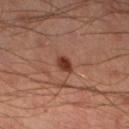workup=catalogued during a skin exam; not biopsied | subject=male, aged 43–47 | diameter=~2 mm (longest diameter) | location=the left lower leg | image source=15 mm crop, total-body photography | illumination=cross-polarized illumination | TBP lesion metrics=an area of roughly 3 mm² and a shape eccentricity near 0.6; a mean CIELAB color near L≈35 a*≈24 b*≈28, roughly 12 lightness units darker than nearby skin, and a normalized lesion–skin contrast near 10.